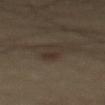This lesion was catalogued during total-body skin photography and was not selected for biopsy. A male patient, aged 58–62. Automated image analysis of the tile measured an outline eccentricity of about 0.9 (0 = round, 1 = elongated) and a shape-asymmetry score of about 0.2 (0 = symmetric). It also reported a mean CIELAB color near L≈30 a*≈9 b*≈20, a lesion–skin lightness drop of about 5, and a normalized border contrast of about 5.5. The software also gave a classifier nevus-likeness of about 5/100. Imaged with cross-polarized lighting. Cropped from a total-body skin-imaging series; the visible field is about 15 mm. Located on the back. The lesion's longest dimension is about 4 mm.